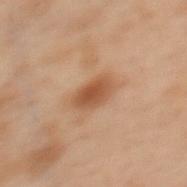Findings:
* notes — imaged on a skin check; not biopsied
* automated metrics — an average lesion color of about L≈44 a*≈19 b*≈29 (CIELAB) and a lesion-to-skin contrast of about 7.5 (normalized; higher = more distinct); a nevus-likeness score of about 60/100 and a lesion-detection confidence of about 100/100
* imaging modality — ~15 mm tile from a whole-body skin photo
* subject — female, in their mid-50s
* body site — the upper back
* lesion size — ~3.5 mm (longest diameter)